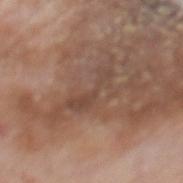– imaging modality — ~15 mm tile from a whole-body skin photo
– automated metrics — a normalized lesion–skin contrast near 5.5; border irregularity of about 8.5 on a 0–10 scale, internal color variation of about 2 on a 0–10 scale, and a peripheral color-asymmetry measure near 0.5; an automated nevus-likeness rating near 0 out of 100 and a detector confidence of about 55 out of 100 that the crop contains a lesion
– anatomic site — the back
– tile lighting — white-light
– subject — male, aged approximately 75
– lesion size — ≈5 mm